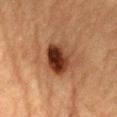Imaged during a routine full-body skin examination; the lesion was not biopsied and no histopathology is available. Captured under cross-polarized illumination. The lesion is located on the abdomen. The lesion-visualizer software estimated an automated nevus-likeness rating near 95 out of 100 and a lesion-detection confidence of about 100/100. A 15 mm close-up extracted from a 3D total-body photography capture. The recorded lesion diameter is about 4 mm. A male subject, aged around 85 or older.